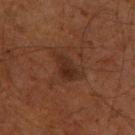Impression:
This lesion was catalogued during total-body skin photography and was not selected for biopsy.
Background:
The patient is a male aged approximately 60. The total-body-photography lesion software estimated an average lesion color of about L≈23 a*≈16 b*≈22 (CIELAB), a lesion–skin lightness drop of about 6, and a normalized border contrast of about 7. The software also gave a nevus-likeness score of about 10/100 and a detector confidence of about 100 out of 100 that the crop contains a lesion. Imaged with cross-polarized lighting. The lesion is located on the left thigh. Longest diameter approximately 4.5 mm. A lesion tile, about 15 mm wide, cut from a 3D total-body photograph.